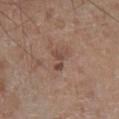No biopsy was performed on this lesion — it was imaged during a full skin examination and was not determined to be concerning. An algorithmic analysis of the crop reported an area of roughly 3.5 mm², a shape eccentricity near 0.8, and a symmetry-axis asymmetry near 0.6. It also reported a border-irregularity rating of about 7/10, internal color variation of about 0.5 on a 0–10 scale, and radial color variation of about 0. The patient is a male aged 58–62. Cropped from a total-body skin-imaging series; the visible field is about 15 mm. Located on the leg. This is a white-light tile. Approximately 3 mm at its widest.